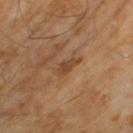No biopsy was performed on this lesion — it was imaged during a full skin examination and was not determined to be concerning.
The recorded lesion diameter is about 3 mm.
The patient is a male aged around 65.
Automated image analysis of the tile measured a lesion area of about 4 mm² and an eccentricity of roughly 0.85. It also reported a lesion color around L≈43 a*≈19 b*≈32 in CIELAB, roughly 8 lightness units darker than nearby skin, and a normalized lesion–skin contrast near 7.
This is a cross-polarized tile.
A region of skin cropped from a whole-body photographic capture, roughly 15 mm wide.